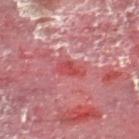  biopsy_status: not biopsied; imaged during a skin examination
  site: right lower leg
  lesion_size:
    long_diameter_mm_approx: 3.0
  automated_metrics:
    border_irregularity_0_10: 3.0
    color_variation_0_10: 2.5
    peripheral_color_asymmetry: 1.0
    lesion_detection_confidence_0_100: 60
  image:
    source: total-body photography crop
    field_of_view_mm: 15
  patient:
    sex: male
    age_approx: 55